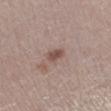A female subject, in their 40s. On the left lower leg. An algorithmic analysis of the crop reported a footprint of about 3.5 mm², a shape eccentricity near 0.8, and two-axis asymmetry of about 0.2. The software also gave roughly 10 lightness units darker than nearby skin. And it measured a color-variation rating of about 2/10. A 15 mm crop from a total-body photograph taken for skin-cancer surveillance. Captured under white-light illumination.